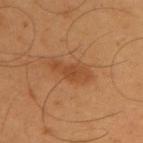Acquisition and patient details:
Imaged with cross-polarized lighting. An algorithmic analysis of the crop reported lesion-presence confidence of about 100/100. A region of skin cropped from a whole-body photographic capture, roughly 15 mm wide. The recorded lesion diameter is about 4.5 mm. A male subject in their mid- to late 50s. From the upper back.